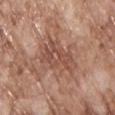| feature | finding |
|---|---|
| notes | imaged on a skin check; not biopsied |
| subject | male, about 70 years old |
| image | ~15 mm tile from a whole-body skin photo |
| anatomic site | the chest |